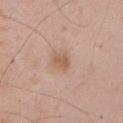workup — catalogued during a skin exam; not biopsied
subject — male, in their mid-40s
location — the left upper arm
imaging modality — 15 mm crop, total-body photography